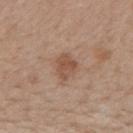Impression:
Recorded during total-body skin imaging; not selected for excision or biopsy.
Background:
A 15 mm close-up tile from a total-body photography series done for melanoma screening. From the mid back. A male patient roughly 65 years of age.